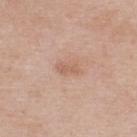Clinical impression:
No biopsy was performed on this lesion — it was imaged during a full skin examination and was not determined to be concerning.
Context:
On the back. The subject is a male aged 58–62. A 15 mm close-up tile from a total-body photography series done for melanoma screening.